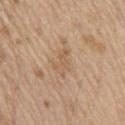Q: Is there a histopathology result?
A: no biopsy performed (imaged during a skin exam)
Q: Patient demographics?
A: male, aged around 70
Q: How was this image acquired?
A: ~15 mm tile from a whole-body skin photo
Q: Lesion location?
A: the mid back
Q: What lighting was used for the tile?
A: white-light illumination
Q: Lesion size?
A: about 3 mm
Q: Automated lesion metrics?
A: an area of roughly 4 mm², a shape eccentricity near 0.9, and a shape-asymmetry score of about 0.45 (0 = symmetric); an average lesion color of about L≈58 a*≈17 b*≈32 (CIELAB) and a lesion-to-skin contrast of about 4.5 (normalized; higher = more distinct)